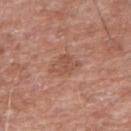Background: Captured under white-light illumination. Measured at roughly 3 mm in maximum diameter. Automated tile analysis of the lesion measured a mean CIELAB color near L≈52 a*≈23 b*≈29, about 7 CIELAB-L* units darker than the surrounding skin, and a normalized border contrast of about 5.5. The software also gave a peripheral color-asymmetry measure near 1. The software also gave an automated nevus-likeness rating near 0 out of 100 and lesion-presence confidence of about 100/100. Located on the right upper arm. A 15 mm close-up tile from a total-body photography series done for melanoma screening. A male patient in their 60s.